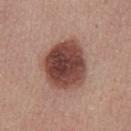| feature | finding |
|---|---|
| follow-up | catalogued during a skin exam; not biopsied |
| imaging modality | ~15 mm tile from a whole-body skin photo |
| TBP lesion metrics | a lesion–skin lightness drop of about 18 and a normalized border contrast of about 12.5; internal color variation of about 5.5 on a 0–10 scale; a classifier nevus-likeness of about 85/100 and lesion-presence confidence of about 100/100 |
| lesion diameter | ≈6.5 mm |
| tile lighting | white-light |
| site | the chest |
| subject | male, aged 23–27 |